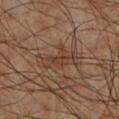Image and clinical context:
Located on the left lower leg. The subject is a male about 60 years old. Cropped from a total-body skin-imaging series; the visible field is about 15 mm.